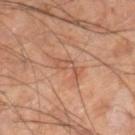– biopsy status: total-body-photography surveillance lesion; no biopsy
– body site: the right lower leg
– subject: male, roughly 60 years of age
– illumination: cross-polarized
– automated metrics: an area of roughly 6.5 mm² and a shape-asymmetry score of about 0.65 (0 = symmetric); a mean CIELAB color near L≈53 a*≈22 b*≈31 and a normalized border contrast of about 5; a classifier nevus-likeness of about 0/100 and a lesion-detection confidence of about 100/100
– acquisition: total-body-photography crop, ~15 mm field of view
– lesion size: ~5 mm (longest diameter)